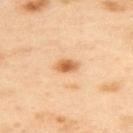biopsy status=catalogued during a skin exam; not biopsied | body site=the upper back | patient=female, about 40 years old | lesion diameter=~3 mm (longest diameter) | lighting=cross-polarized illumination | image=total-body-photography crop, ~15 mm field of view | TBP lesion metrics=an area of roughly 4 mm², an eccentricity of roughly 0.85, and two-axis asymmetry of about 0.15; a lesion color around L≈66 a*≈25 b*≈43 in CIELAB, roughly 14 lightness units darker than nearby skin, and a normalized lesion–skin contrast near 9; a border-irregularity index near 1.5/10 and peripheral color asymmetry of about 1.5.